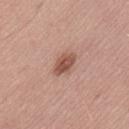Notes:
• body site — the lower back
• subject — male, roughly 55 years of age
• diameter — ≈3 mm
• automated lesion analysis — an area of roughly 5 mm², an outline eccentricity of about 0.75 (0 = round, 1 = elongated), and two-axis asymmetry of about 0.2; roughly 12 lightness units darker than nearby skin and a normalized lesion–skin contrast near 8.5; border irregularity of about 2 on a 0–10 scale and radial color variation of about 1
• image — 15 mm crop, total-body photography
• tile lighting — white-light